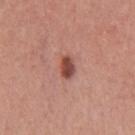Assessment: This lesion was catalogued during total-body skin photography and was not selected for biopsy. Context: About 2.5 mm across. A male subject, approximately 45 years of age. The lesion is located on the mid back. A roughly 15 mm field-of-view crop from a total-body skin photograph. Automated tile analysis of the lesion measured about 15 CIELAB-L* units darker than the surrounding skin and a normalized border contrast of about 10.5.